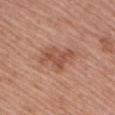notes: imaged on a skin check; not biopsied
lighting: white-light illumination
location: the right upper arm
automated metrics: a footprint of about 8.5 mm² and a symmetry-axis asymmetry near 0.4; a lesion color around L≈52 a*≈24 b*≈30 in CIELAB; a classifier nevus-likeness of about 25/100 and lesion-presence confidence of about 100/100
patient: female, in their mid-60s
image source: total-body-photography crop, ~15 mm field of view
lesion diameter: ~4.5 mm (longest diameter)